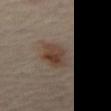| field | value |
|---|---|
| image | ~15 mm tile from a whole-body skin photo |
| patient | about 55 years old |
| body site | the abdomen |
| image-analysis metrics | a lesion color around L≈39 a*≈14 b*≈23 in CIELAB, roughly 9 lightness units darker than nearby skin, and a lesion-to-skin contrast of about 8 (normalized; higher = more distinct); a border-irregularity index near 1.5/10, internal color variation of about 5.5 on a 0–10 scale, and a peripheral color-asymmetry measure near 1.5; a nevus-likeness score of about 90/100 and lesion-presence confidence of about 100/100 |
| lesion size | ~4.5 mm (longest diameter) |